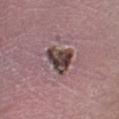notes: imaged on a skin check; not biopsied | size: ≈4 mm | body site: the left lower leg | imaging modality: total-body-photography crop, ~15 mm field of view | lighting: white-light illumination | subject: male, approximately 55 years of age.